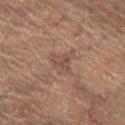This lesion was catalogued during total-body skin photography and was not selected for biopsy. A male subject, in their mid-60s. Captured under cross-polarized illumination. This image is a 15 mm lesion crop taken from a total-body photograph. The lesion's longest dimension is about 3 mm. Located on the right lower leg. Automated image analysis of the tile measured a footprint of about 5 mm², an outline eccentricity of about 0.4 (0 = round, 1 = elongated), and a shape-asymmetry score of about 0.5 (0 = symmetric). And it measured a lesion-to-skin contrast of about 5.5 (normalized; higher = more distinct).